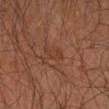Case summary:
* follow-up — no biopsy performed (imaged during a skin exam)
* acquisition — total-body-photography crop, ~15 mm field of view
* TBP lesion metrics — an area of roughly 3.5 mm², a shape eccentricity near 0.9, and a symmetry-axis asymmetry near 0.35; a lesion color around L≈37 a*≈22 b*≈30 in CIELAB, a lesion–skin lightness drop of about 5, and a normalized border contrast of about 4.5; a border-irregularity index near 3.5/10, a within-lesion color-variation index near 1/10, and peripheral color asymmetry of about 0.5
* diameter — ≈3 mm
* anatomic site — the arm
* patient — male, approximately 55 years of age